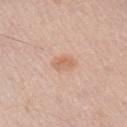The lesion was tiled from a total-body skin photograph and was not biopsied. A 15 mm crop from a total-body photograph taken for skin-cancer surveillance. The lesion is on the right upper arm. A male subject, aged approximately 50. About 2.5 mm across.